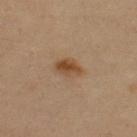Q: Was a biopsy performed?
A: catalogued during a skin exam; not biopsied
Q: Lesion size?
A: ≈3.5 mm
Q: How was the tile lit?
A: cross-polarized
Q: Patient demographics?
A: male, in their mid-30s
Q: What is the imaging modality?
A: ~15 mm crop, total-body skin-cancer survey
Q: Lesion location?
A: the back